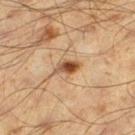On the left thigh.
A male subject about 60 years old.
A roughly 15 mm field-of-view crop from a total-body skin photograph.
This is a cross-polarized tile.
Measured at roughly 3 mm in maximum diameter.